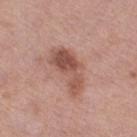Clinical impression: Recorded during total-body skin imaging; not selected for excision or biopsy. Background: The subject is a female aged 38–42. The lesion-visualizer software estimated an area of roughly 13 mm² and two-axis asymmetry of about 0.45. The analysis additionally found a mean CIELAB color near L≈52 a*≈23 b*≈26, a lesion–skin lightness drop of about 11, and a lesion-to-skin contrast of about 8 (normalized; higher = more distinct). The software also gave a border-irregularity index near 5/10, a color-variation rating of about 6.5/10, and radial color variation of about 2.5. And it measured a classifier nevus-likeness of about 40/100 and lesion-presence confidence of about 100/100. Cropped from a total-body skin-imaging series; the visible field is about 15 mm. The tile uses white-light illumination. The lesion is located on the right thigh.